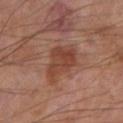The lesion was photographed on a routine skin check and not biopsied; there is no pathology result.
The total-body-photography lesion software estimated an area of roughly 13 mm² and an eccentricity of roughly 0.75. And it measured border irregularity of about 3 on a 0–10 scale and a peripheral color-asymmetry measure near 1.
A roughly 15 mm field-of-view crop from a total-body skin photograph.
The tile uses cross-polarized illumination.
From the leg.
The patient is a male in their 70s.